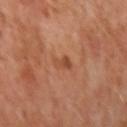The lesion was tiled from a total-body skin photograph and was not biopsied. A female subject in their 50s. Located on the left forearm. This image is a 15 mm lesion crop taken from a total-body photograph. The recorded lesion diameter is about 2.5 mm. This is a cross-polarized tile. An algorithmic analysis of the crop reported an outline eccentricity of about 0.9 (0 = round, 1 = elongated). The analysis additionally found a border-irregularity rating of about 4.5/10 and a color-variation rating of about 1/10.